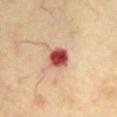notes: catalogued during a skin exam; not biopsied
diameter: ~3 mm (longest diameter)
location: the front of the torso
acquisition: 15 mm crop, total-body photography
subject: female, roughly 60 years of age
lighting: cross-polarized